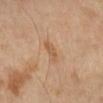follow-up = catalogued during a skin exam; not biopsied | lesion diameter = ~3 mm (longest diameter) | subject = female, aged 68–72 | image source = ~15 mm tile from a whole-body skin photo | TBP lesion metrics = an average lesion color of about L≈58 a*≈20 b*≈36 (CIELAB), roughly 8 lightness units darker than nearby skin, and a normalized border contrast of about 6; a nevus-likeness score of about 5/100 and a detector confidence of about 100 out of 100 that the crop contains a lesion | site = the right lower leg | lighting = cross-polarized.